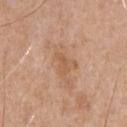Acquisition and patient details: The patient is a male aged approximately 65. An algorithmic analysis of the crop reported a color-variation rating of about 0/10 and radial color variation of about 0. This is a white-light tile. A 15 mm close-up tile from a total-body photography series done for melanoma screening. Located on the chest. Measured at roughly 2.5 mm in maximum diameter.